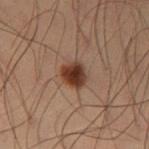follow-up = catalogued during a skin exam; not biopsied
body site = the left thigh
image = 15 mm crop, total-body photography
subject = male, aged 53–57